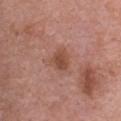Case summary:
– follow-up — imaged on a skin check; not biopsied
– patient — female, aged 63–67
– image-analysis metrics — a lesion area of about 5.5 mm², a shape eccentricity near 0.35, and two-axis asymmetry of about 0.25; a border-irregularity index near 2/10, a within-lesion color-variation index near 3/10, and radial color variation of about 1
– tile lighting — white-light
– diameter — ≈2.5 mm
– anatomic site — the upper back
– imaging modality — 15 mm crop, total-body photography